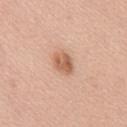No biopsy was performed on this lesion — it was imaged during a full skin examination and was not determined to be concerning.
The subject is a female about 65 years old.
The lesion is located on the chest.
A 15 mm crop from a total-body photograph taken for skin-cancer surveillance.
The tile uses white-light illumination.